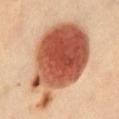The lesion-visualizer software estimated a footprint of about 55 mm², an outline eccentricity of about 0.75 (0 = round, 1 = elongated), and a symmetry-axis asymmetry near 0.25. And it measured a lesion–skin lightness drop of about 22 and a lesion-to-skin contrast of about 13 (normalized; higher = more distinct). Measured at roughly 11 mm in maximum diameter. On the abdomen. A female subject, approximately 60 years of age. A lesion tile, about 15 mm wide, cut from a 3D total-body photograph. The tile uses cross-polarized illumination.